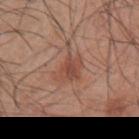  lesion_size:
    long_diameter_mm_approx: 4.0
  image:
    source: total-body photography crop
    field_of_view_mm: 15
  lighting: white-light
  site: left upper arm
  patient:
    sex: male
    age_approx: 45